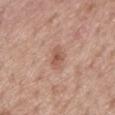Imaged during a routine full-body skin examination; the lesion was not biopsied and no histopathology is available.
Automated tile analysis of the lesion measured a footprint of about 4.5 mm² and a shape-asymmetry score of about 0.2 (0 = symmetric). The software also gave a border-irregularity rating of about 2.5/10, a within-lesion color-variation index near 3/10, and peripheral color asymmetry of about 1. It also reported a classifier nevus-likeness of about 0/100 and lesion-presence confidence of about 100/100.
Located on the mid back.
This image is a 15 mm lesion crop taken from a total-body photograph.
A male patient, in their mid- to late 50s.
About 3 mm across.